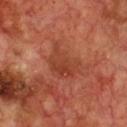Captured during whole-body skin photography for melanoma surveillance; the lesion was not biopsied. Measured at roughly 5 mm in maximum diameter. The lesion is located on the chest. The tile uses cross-polarized illumination. A male patient, aged 68 to 72. Cropped from a total-body skin-imaging series; the visible field is about 15 mm.